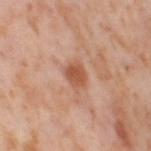Q: Is there a histopathology result?
A: total-body-photography surveillance lesion; no biopsy
Q: What is the lesion's diameter?
A: ~3 mm (longest diameter)
Q: How was the tile lit?
A: cross-polarized
Q: What did automated image analysis measure?
A: an average lesion color of about L≈55 a*≈25 b*≈33 (CIELAB) and a normalized lesion–skin contrast near 7.5; a border-irregularity rating of about 2/10, a color-variation rating of about 3/10, and peripheral color asymmetry of about 1
Q: What is the imaging modality?
A: ~15 mm tile from a whole-body skin photo
Q: What are the patient's age and sex?
A: female, aged around 55
Q: What is the anatomic site?
A: the right thigh